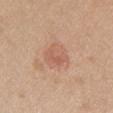Q: Is there a histopathology result?
A: total-body-photography surveillance lesion; no biopsy
Q: What is the imaging modality?
A: 15 mm crop, total-body photography
Q: What is the anatomic site?
A: the front of the torso
Q: How was the tile lit?
A: white-light
Q: Who is the patient?
A: female, aged 38–42
Q: What did automated image analysis measure?
A: an area of roughly 8.5 mm², an eccentricity of roughly 0.6, and a shape-asymmetry score of about 0.2 (0 = symmetric); a nevus-likeness score of about 10/100 and a detector confidence of about 100 out of 100 that the crop contains a lesion